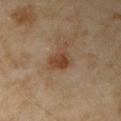notes: total-body-photography surveillance lesion; no biopsy | subject: female, in their mid-30s | automated metrics: an area of roughly 5.5 mm², an outline eccentricity of about 0.65 (0 = round, 1 = elongated), and a shape-asymmetry score of about 0.25 (0 = symmetric); about 9 CIELAB-L* units darker than the surrounding skin and a lesion-to-skin contrast of about 8 (normalized; higher = more distinct); a classifier nevus-likeness of about 55/100 and a detector confidence of about 100 out of 100 that the crop contains a lesion | body site: the right upper arm | imaging modality: ~15 mm crop, total-body skin-cancer survey | tile lighting: cross-polarized.